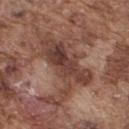notes — imaged on a skin check; not biopsied
acquisition — ~15 mm tile from a whole-body skin photo
diameter — ~7 mm (longest diameter)
body site — the upper back
patient — male, approximately 75 years of age
lighting — white-light illumination